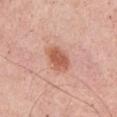This lesion was catalogued during total-body skin photography and was not selected for biopsy. Measured at roughly 3.5 mm in maximum diameter. The subject is a male aged around 55. The lesion is located on the chest. A 15 mm crop from a total-body photograph taken for skin-cancer surveillance.